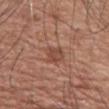Q: Was a biopsy performed?
A: no biopsy performed (imaged during a skin exam)
Q: Illumination type?
A: white-light illumination
Q: Lesion location?
A: the chest
Q: What kind of image is this?
A: 15 mm crop, total-body photography
Q: How large is the lesion?
A: ~3 mm (longest diameter)
Q: Who is the patient?
A: male, aged around 70